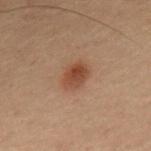{
  "biopsy_status": "not biopsied; imaged during a skin examination",
  "site": "mid back",
  "patient": {
    "sex": "male",
    "age_approx": 55
  },
  "lighting": "cross-polarized",
  "image": {
    "source": "total-body photography crop",
    "field_of_view_mm": 15
  },
  "lesion_size": {
    "long_diameter_mm_approx": 3.5
  }
}